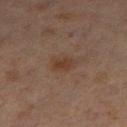follow-up = catalogued during a skin exam; not biopsied | location = the left thigh | subject = male, roughly 60 years of age | lesion size = ≈2.5 mm | image-analysis metrics = an area of roughly 4 mm² and two-axis asymmetry of about 0.25; border irregularity of about 2 on a 0–10 scale, a within-lesion color-variation index near 2.5/10, and a peripheral color-asymmetry measure near 1 | illumination = cross-polarized | acquisition = ~15 mm crop, total-body skin-cancer survey.